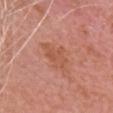Notes:
- biopsy status · catalogued during a skin exam; not biopsied
- patient · male, approximately 75 years of age
- acquisition · 15 mm crop, total-body photography
- anatomic site · the head or neck